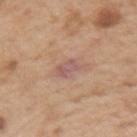No biopsy was performed on this lesion — it was imaged during a full skin examination and was not determined to be concerning. Longest diameter approximately 3.5 mm. The total-body-photography lesion software estimated a mean CIELAB color near L≈57 a*≈20 b*≈24, about 7 CIELAB-L* units darker than the surrounding skin, and a normalized border contrast of about 6.5. Imaged with white-light lighting. A 15 mm close-up tile from a total-body photography series done for melanoma screening. The patient is a male roughly 70 years of age. The lesion is located on the right upper arm.